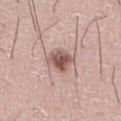Q: Was this lesion biopsied?
A: catalogued during a skin exam; not biopsied
Q: Who is the patient?
A: male, roughly 65 years of age
Q: What kind of image is this?
A: total-body-photography crop, ~15 mm field of view
Q: What is the anatomic site?
A: the abdomen